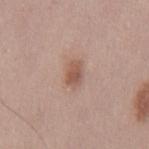workup = imaged on a skin check; not biopsied.